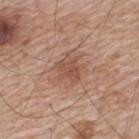Located on the upper back. A region of skin cropped from a whole-body photographic capture, roughly 15 mm wide. This is a white-light tile. An algorithmic analysis of the crop reported an outline eccentricity of about 0.35 (0 = round, 1 = elongated) and a symmetry-axis asymmetry near 0.3. The analysis additionally found a mean CIELAB color near L≈51 a*≈21 b*≈28 and a lesion-to-skin contrast of about 6 (normalized; higher = more distinct). And it measured border irregularity of about 3.5 on a 0–10 scale and a peripheral color-asymmetry measure near 0.5. A male subject aged approximately 65. Measured at roughly 3 mm in maximum diameter.